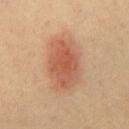{
  "biopsy_status": "not biopsied; imaged during a skin examination",
  "site": "chest",
  "automated_metrics": {
    "eccentricity": 0.8,
    "shape_asymmetry": 0.15,
    "vs_skin_darker_L": 11.0,
    "vs_skin_contrast_norm": 7.5,
    "color_variation_0_10": 4.5,
    "peripheral_color_asymmetry": 1.5,
    "nevus_likeness_0_100": 100,
    "lesion_detection_confidence_0_100": 100
  },
  "image": {
    "source": "total-body photography crop",
    "field_of_view_mm": 15
  },
  "lighting": "cross-polarized",
  "patient": {
    "sex": "male",
    "age_approx": 40
  },
  "lesion_size": {
    "long_diameter_mm_approx": 6.0
  }
}